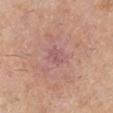Imaged during a routine full-body skin examination; the lesion was not biopsied and no histopathology is available.
A region of skin cropped from a whole-body photographic capture, roughly 15 mm wide.
The lesion's longest dimension is about 2.5 mm.
Located on the left lower leg.
The total-body-photography lesion software estimated a border-irregularity rating of about 2.5/10, a within-lesion color-variation index near 2/10, and radial color variation of about 0.5. The analysis additionally found a nevus-likeness score of about 0/100.
A female patient aged around 50.
This is a cross-polarized tile.